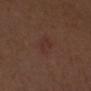Approximately 3 mm at its widest. A lesion tile, about 15 mm wide, cut from a 3D total-body photograph. Automated image analysis of the tile measured a border-irregularity rating of about 3/10, internal color variation of about 1.5 on a 0–10 scale, and radial color variation of about 0.5. It also reported lesion-presence confidence of about 100/100. A female subject, roughly 30 years of age. From the chest. On excision, pathology confirmed an actinic keratosis (borderline).